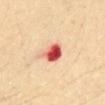Captured during whole-body skin photography for melanoma surveillance; the lesion was not biopsied.